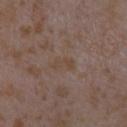Assessment:
Captured during whole-body skin photography for melanoma surveillance; the lesion was not biopsied.
Clinical summary:
The subject is a female approximately 35 years of age. A region of skin cropped from a whole-body photographic capture, roughly 15 mm wide. Located on the right forearm.